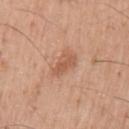follow-up = total-body-photography surveillance lesion; no biopsy | diameter = ~4 mm (longest diameter) | image-analysis metrics = a lesion area of about 6 mm², a shape eccentricity near 0.85, and two-axis asymmetry of about 0.3; border irregularity of about 3 on a 0–10 scale, internal color variation of about 2 on a 0–10 scale, and peripheral color asymmetry of about 1; a lesion-detection confidence of about 100/100 | imaging modality = 15 mm crop, total-body photography | body site = the arm | lighting = white-light illumination | patient = male, roughly 55 years of age.